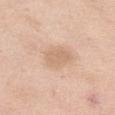A 15 mm crop from a total-body photograph taken for skin-cancer surveillance.
A female subject aged 53 to 57.
The lesion's longest dimension is about 3.5 mm.
This is a white-light tile.
Located on the left thigh.